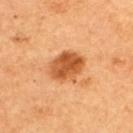Notes:
– lighting — cross-polarized illumination
– diameter — ~4 mm (longest diameter)
– subject — male, about 60 years old
– TBP lesion metrics — an automated nevus-likeness rating near 95 out of 100 and lesion-presence confidence of about 100/100
– body site — the upper back
– image source — ~15 mm crop, total-body skin-cancer survey